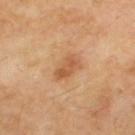No biopsy was performed on this lesion — it was imaged during a full skin examination and was not determined to be concerning.